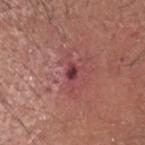The patient is a male aged approximately 65.
The lesion is located on the head or neck.
Cropped from a total-body skin-imaging series; the visible field is about 15 mm.
The biopsy diagnosis was a skin cancer: invasive squamous cell carcinoma.